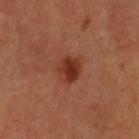Recorded during total-body skin imaging; not selected for excision or biopsy.
The lesion is on the chest.
A lesion tile, about 15 mm wide, cut from a 3D total-body photograph.
A female patient, roughly 50 years of age.